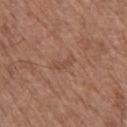| key | value |
|---|---|
| notes | total-body-photography surveillance lesion; no biopsy |
| lesion size | ~3 mm (longest diameter) |
| body site | the upper back |
| imaging modality | total-body-photography crop, ~15 mm field of view |
| subject | male, in their mid- to late 70s |